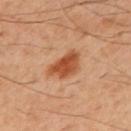biopsy status: imaged on a skin check; not biopsied | anatomic site: the mid back | acquisition: ~15 mm tile from a whole-body skin photo | patient: male, in their mid-40s | lesion size: ≈4.5 mm.